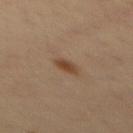No biopsy was performed on this lesion — it was imaged during a full skin examination and was not determined to be concerning. This is a cross-polarized tile. Located on the back. A male subject, aged around 35. Cropped from a total-body skin-imaging series; the visible field is about 15 mm. About 3 mm across. An algorithmic analysis of the crop reported a classifier nevus-likeness of about 85/100 and a lesion-detection confidence of about 100/100.